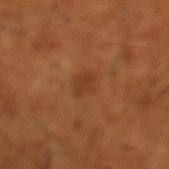Q: How large is the lesion?
A: ~2.5 mm (longest diameter)
Q: What kind of image is this?
A: ~15 mm crop, total-body skin-cancer survey
Q: What is the anatomic site?
A: the left lower leg
Q: What are the patient's age and sex?
A: male, approximately 65 years of age